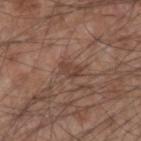  biopsy_status: not biopsied; imaged during a skin examination
  image:
    source: total-body photography crop
    field_of_view_mm: 15
  lighting: white-light
  lesion_size:
    long_diameter_mm_approx: 2.5
  patient:
    sex: male
    age_approx: 55
  site: left forearm